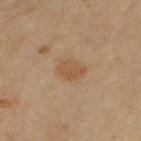workup = imaged on a skin check; not biopsied | automated lesion analysis = a lesion color around L≈52 a*≈18 b*≈34 in CIELAB and about 7 CIELAB-L* units darker than the surrounding skin; a color-variation rating of about 1.5/10 and a peripheral color-asymmetry measure near 0.5 | body site = the left upper arm | size = ~3 mm (longest diameter) | patient = female, approximately 60 years of age | illumination = cross-polarized illumination | imaging modality = ~15 mm tile from a whole-body skin photo.